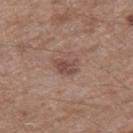Part of a total-body skin-imaging series; this lesion was reviewed on a skin check and was not flagged for biopsy.
Captured under white-light illumination.
Approximately 3 mm at its widest.
A lesion tile, about 15 mm wide, cut from a 3D total-body photograph.
Automated tile analysis of the lesion measured an eccentricity of roughly 0.7 and two-axis asymmetry of about 0.4. It also reported a nevus-likeness score of about 10/100 and a lesion-detection confidence of about 100/100.
The patient is a male aged 63 to 67.
From the right thigh.